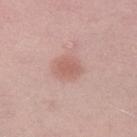No biopsy was performed on this lesion — it was imaged during a full skin examination and was not determined to be concerning. Cropped from a whole-body photographic skin survey; the tile spans about 15 mm. From the right thigh. A female subject, aged around 20.